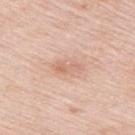* notes: no biopsy performed (imaged during a skin exam)
* tile lighting: white-light
* patient: female, about 65 years old
* diameter: about 3.5 mm
* image source: 15 mm crop, total-body photography
* body site: the right upper arm